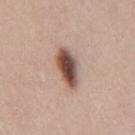notes: total-body-photography surveillance lesion; no biopsy | patient: male, in their mid- to late 40s | imaging modality: total-body-photography crop, ~15 mm field of view | lesion diameter: ~5 mm (longest diameter) | body site: the mid back | automated metrics: a footprint of about 9.5 mm² and an outline eccentricity of about 0.85 (0 = round, 1 = elongated); a nevus-likeness score of about 100/100 and a lesion-detection confidence of about 100/100.